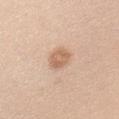The lesion was photographed on a routine skin check and not biopsied; there is no pathology result.
On the left upper arm.
The lesion-visualizer software estimated an area of roughly 6 mm² and a symmetry-axis asymmetry near 0.2. It also reported a lesion color around L≈64 a*≈19 b*≈32 in CIELAB, a lesion–skin lightness drop of about 10, and a normalized border contrast of about 7. The software also gave a border-irregularity index near 2/10, a within-lesion color-variation index near 3/10, and a peripheral color-asymmetry measure near 1.
The lesion's longest dimension is about 3.5 mm.
The patient is a female aged 23 to 27.
Cropped from a whole-body photographic skin survey; the tile spans about 15 mm.
The tile uses white-light illumination.